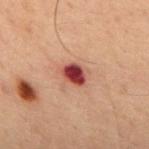Captured during whole-body skin photography for melanoma surveillance; the lesion was not biopsied.
From the back.
A male subject, in their 60s.
This is a cross-polarized tile.
Approximately 2.5 mm at its widest.
Cropped from a total-body skin-imaging series; the visible field is about 15 mm.
The lesion-visualizer software estimated a classifier nevus-likeness of about 0/100 and a detector confidence of about 100 out of 100 that the crop contains a lesion.